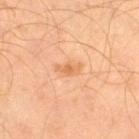Q: Was this lesion biopsied?
A: imaged on a skin check; not biopsied
Q: Lesion size?
A: ≈3 mm
Q: Lesion location?
A: the right thigh
Q: What lighting was used for the tile?
A: cross-polarized
Q: How was this image acquired?
A: 15 mm crop, total-body photography
Q: Automated lesion metrics?
A: an area of roughly 3 mm², an outline eccentricity of about 0.85 (0 = round, 1 = elongated), and a symmetry-axis asymmetry near 0.3; a mean CIELAB color near L≈63 a*≈23 b*≈40, roughly 8 lightness units darker than nearby skin, and a lesion-to-skin contrast of about 6 (normalized; higher = more distinct)
Q: What are the patient's age and sex?
A: male, aged 58 to 62